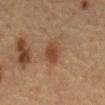<tbp_lesion>
  <biopsy_status>not biopsied; imaged during a skin examination</biopsy_status>
  <image>
    <source>total-body photography crop</source>
    <field_of_view_mm>15</field_of_view_mm>
  </image>
  <lesion_size>
    <long_diameter_mm_approx>3.5</long_diameter_mm_approx>
  </lesion_size>
  <site>abdomen</site>
  <patient>
    <sex>male</sex>
    <age_approx>65</age_approx>
  </patient>
</tbp_lesion>